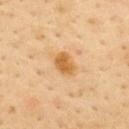Assessment: Captured during whole-body skin photography for melanoma surveillance; the lesion was not biopsied. Image and clinical context: The subject is a female in their 50s. Captured under cross-polarized illumination. This image is a 15 mm lesion crop taken from a total-body photograph. The lesion is located on the upper back.